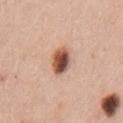Background:
This is a white-light tile. The patient is a female aged approximately 45. On the left upper arm. The total-body-photography lesion software estimated a lesion area of about 7 mm² and an outline eccentricity of about 0.65 (0 = round, 1 = elongated). It also reported a nevus-likeness score of about 100/100 and lesion-presence confidence of about 100/100. A roughly 15 mm field-of-view crop from a total-body skin photograph. Longest diameter approximately 3.5 mm.